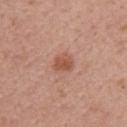Case summary:
– biopsy status · total-body-photography surveillance lesion; no biopsy
– size · ≈2.5 mm
– imaging modality · 15 mm crop, total-body photography
– anatomic site · the right upper arm
– patient · female, approximately 50 years of age